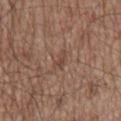Imaged during a routine full-body skin examination; the lesion was not biopsied and no histopathology is available. Approximately 2.5 mm at its widest. The total-body-photography lesion software estimated a classifier nevus-likeness of about 0/100 and a detector confidence of about 75 out of 100 that the crop contains a lesion. The tile uses white-light illumination. A male patient roughly 75 years of age. The lesion is located on the mid back. A 15 mm crop from a total-body photograph taken for skin-cancer surveillance.